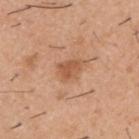Case summary:
– workup: no biopsy performed (imaged during a skin exam)
– diameter: ≈3 mm
– image-analysis metrics: an outline eccentricity of about 0.7 (0 = round, 1 = elongated); a mean CIELAB color near L≈56 a*≈23 b*≈35 and a normalized border contrast of about 6.5; a border-irregularity index near 3.5/10, internal color variation of about 2 on a 0–10 scale, and radial color variation of about 0.5; a classifier nevus-likeness of about 5/100 and a detector confidence of about 100 out of 100 that the crop contains a lesion
– lighting: white-light
– body site: the back
– acquisition: ~15 mm crop, total-body skin-cancer survey
– patient: male, approximately 40 years of age